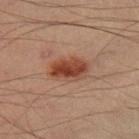workup — total-body-photography surveillance lesion; no biopsy | automated metrics — a mean CIELAB color near L≈39 a*≈22 b*≈27, about 11 CIELAB-L* units darker than the surrounding skin, and a normalized border contrast of about 9.5; border irregularity of about 2 on a 0–10 scale and radial color variation of about 1.5 | size — ~4.5 mm (longest diameter) | image source — ~15 mm crop, total-body skin-cancer survey | subject — male, aged approximately 40 | body site — the right thigh.